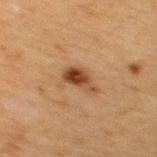Q: How large is the lesion?
A: ≈3.5 mm
Q: What lighting was used for the tile?
A: cross-polarized illumination
Q: Patient demographics?
A: male, aged 48–52
Q: How was this image acquired?
A: ~15 mm tile from a whole-body skin photo
Q: Where on the body is the lesion?
A: the upper back